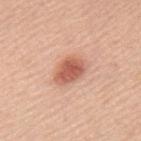Notes:
– notes: no biopsy performed (imaged during a skin exam)
– image source: total-body-photography crop, ~15 mm field of view
– lighting: white-light
– diameter: about 4 mm
– patient: female, in their mid-60s
– location: the back
– automated lesion analysis: a lesion area of about 8 mm² and two-axis asymmetry of about 0.15; border irregularity of about 1.5 on a 0–10 scale and a color-variation rating of about 3/10